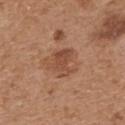Case summary:
* patient · male, in their 70s
* body site · the upper back
* lighting · white-light illumination
* acquisition · 15 mm crop, total-body photography